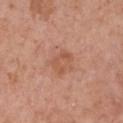Part of a total-body skin-imaging series; this lesion was reviewed on a skin check and was not flagged for biopsy.
Longest diameter approximately 3 mm.
The lesion-visualizer software estimated a normalized border contrast of about 5.5. The analysis additionally found border irregularity of about 7.5 on a 0–10 scale, a color-variation rating of about 0/10, and radial color variation of about 0. And it measured lesion-presence confidence of about 100/100.
Imaged with white-light lighting.
On the chest.
A female subject, aged 58 to 62.
Cropped from a total-body skin-imaging series; the visible field is about 15 mm.